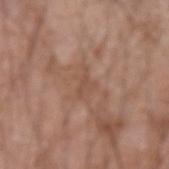The lesion was tiled from a total-body skin photograph and was not biopsied. Longest diameter approximately 3 mm. Imaged with white-light lighting. The total-body-photography lesion software estimated a border-irregularity rating of about 7/10, a within-lesion color-variation index near 0/10, and radial color variation of about 0. A region of skin cropped from a whole-body photographic capture, roughly 15 mm wide. Located on the left forearm. A male patient aged 58–62.